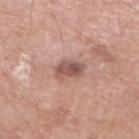Assessment: Captured during whole-body skin photography for melanoma surveillance; the lesion was not biopsied. Clinical summary: On the left lower leg. Longest diameter approximately 3.5 mm. An algorithmic analysis of the crop reported a mean CIELAB color near L≈54 a*≈21 b*≈25 and a normalized lesion–skin contrast near 8.5. Cropped from a whole-body photographic skin survey; the tile spans about 15 mm. A male patient aged approximately 80.